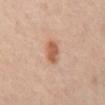No biopsy was performed on this lesion — it was imaged during a full skin examination and was not determined to be concerning.
The tile uses cross-polarized illumination.
This image is a 15 mm lesion crop taken from a total-body photograph.
A male patient, in their mid- to late 60s.
Approximately 3 mm at its widest.
Located on the abdomen.
The lesion-visualizer software estimated an area of roughly 5.5 mm² and two-axis asymmetry of about 0.2. It also reported an average lesion color of about L≈58 a*≈23 b*≈33 (CIELAB), a lesion–skin lightness drop of about 11, and a normalized lesion–skin contrast near 8. And it measured lesion-presence confidence of about 100/100.